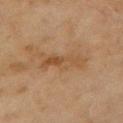Recorded during total-body skin imaging; not selected for excision or biopsy.
The subject is a female approximately 60 years of age.
Located on the left upper arm.
Imaged with cross-polarized lighting.
A 15 mm close-up tile from a total-body photography series done for melanoma screening.